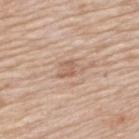biopsy status = no biopsy performed (imaged during a skin exam).